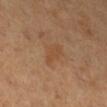follow-up: total-body-photography surveillance lesion; no biopsy
TBP lesion metrics: a lesion area of about 5.5 mm², an eccentricity of roughly 0.75, and a shape-asymmetry score of about 0.3 (0 = symmetric); an automated nevus-likeness rating near 5 out of 100 and a lesion-detection confidence of about 100/100
patient: female, roughly 30 years of age
lesion diameter: about 3.5 mm
imaging modality: ~15 mm crop, total-body skin-cancer survey
tile lighting: cross-polarized
site: the right lower leg